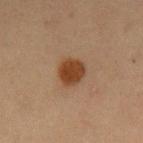Impression: Part of a total-body skin-imaging series; this lesion was reviewed on a skin check and was not flagged for biopsy. Clinical summary: A male subject, aged approximately 60. Measured at roughly 3.5 mm in maximum diameter. The total-body-photography lesion software estimated a footprint of about 7 mm², a shape eccentricity near 0.55, and a symmetry-axis asymmetry near 0.2. The software also gave a mean CIELAB color near L≈34 a*≈19 b*≈29 and roughly 11 lightness units darker than nearby skin. It also reported border irregularity of about 2 on a 0–10 scale and radial color variation of about 0.5. The software also gave a classifier nevus-likeness of about 100/100 and a detector confidence of about 100 out of 100 that the crop contains a lesion. The lesion is on the left upper arm. A region of skin cropped from a whole-body photographic capture, roughly 15 mm wide. Captured under cross-polarized illumination.